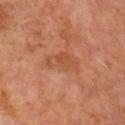biopsy_status: not biopsied; imaged during a skin examination
image:
  source: total-body photography crop
  field_of_view_mm: 15
lesion_size:
  long_diameter_mm_approx: 4.0
site: right upper arm
automated_metrics:
  area_mm2_approx: 7.5
  shape_asymmetry: 0.3
  vs_skin_darker_L: 6.0
  vs_skin_contrast_norm: 5.0
patient:
  sex: male
  age_approx: 65